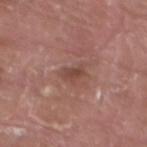workup — total-body-photography surveillance lesion; no biopsy
image source — 15 mm crop, total-body photography
diameter — ~2.5 mm (longest diameter)
illumination — white-light
subject — male, about 40 years old
anatomic site — the leg